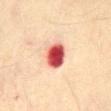{
  "biopsy_status": "not biopsied; imaged during a skin examination",
  "patient": {
    "sex": "male",
    "age_approx": 75
  },
  "automated_metrics": {
    "area_mm2_approx": 8.5,
    "eccentricity": 0.55,
    "shape_asymmetry": 0.15,
    "cielab_L": 48,
    "cielab_a": 34,
    "cielab_b": 27,
    "vs_skin_darker_L": 22.0,
    "vs_skin_contrast_norm": 14.5
  },
  "lighting": "cross-polarized",
  "image": {
    "source": "total-body photography crop",
    "field_of_view_mm": 15
  },
  "lesion_size": {
    "long_diameter_mm_approx": 3.5
  },
  "site": "chest"
}